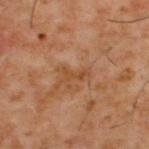Assessment:
The lesion was tiled from a total-body skin photograph and was not biopsied.
Context:
A male patient aged approximately 60. Imaged with cross-polarized lighting. The lesion is on the upper back. About 3 mm across. This image is a 15 mm lesion crop taken from a total-body photograph.